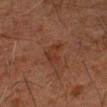follow-up: no biopsy performed (imaged during a skin exam); patient: male, aged approximately 60; tile lighting: cross-polarized illumination; imaging modality: total-body-photography crop, ~15 mm field of view; body site: the left arm.